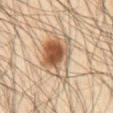Captured during whole-body skin photography for melanoma surveillance; the lesion was not biopsied. A male subject roughly 65 years of age. Automated image analysis of the tile measured a border-irregularity rating of about 3/10, internal color variation of about 9.5 on a 0–10 scale, and radial color variation of about 3. And it measured a lesion-detection confidence of about 100/100. A 15 mm close-up tile from a total-body photography series done for melanoma screening. This is a cross-polarized tile. On the front of the torso.